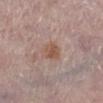The lesion was tiled from a total-body skin photograph and was not biopsied.
The subject is a female aged 63–67.
A 15 mm close-up extracted from a 3D total-body photography capture.
Located on the left lower leg.